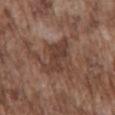The lesion was tiled from a total-body skin photograph and was not biopsied. Captured under white-light illumination. The lesion's longest dimension is about 5.5 mm. Automated image analysis of the tile measured a border-irregularity index near 4/10, internal color variation of about 4 on a 0–10 scale, and a peripheral color-asymmetry measure near 1. The analysis additionally found a nevus-likeness score of about 0/100. A male patient aged approximately 75. From the back. This image is a 15 mm lesion crop taken from a total-body photograph.